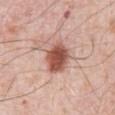Notes:
– workup: total-body-photography surveillance lesion; no biopsy
– site: the front of the torso
– image source: total-body-photography crop, ~15 mm field of view
– subject: male, about 80 years old